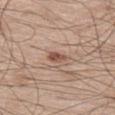{
  "biopsy_status": "not biopsied; imaged during a skin examination",
  "image": {
    "source": "total-body photography crop",
    "field_of_view_mm": 15
  },
  "patient": {
    "sex": "male",
    "age_approx": 60
  },
  "lighting": "white-light",
  "site": "left thigh"
}